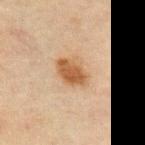Recorded during total-body skin imaging; not selected for excision or biopsy.
The lesion is located on the abdomen.
Captured under cross-polarized illumination.
A lesion tile, about 15 mm wide, cut from a 3D total-body photograph.
The patient is a male aged 68–72.